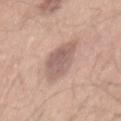| field | value |
|---|---|
| body site | the back |
| acquisition | ~15 mm crop, total-body skin-cancer survey |
| subject | male, about 30 years old |
| automated lesion analysis | an area of roughly 13 mm², a shape eccentricity near 0.9, and two-axis asymmetry of about 0.2; a color-variation rating of about 3.5/10 and radial color variation of about 1 |